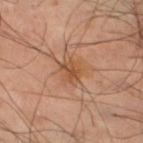<case>
  <biopsy_status>not biopsied; imaged during a skin examination</biopsy_status>
  <lesion_size>
    <long_diameter_mm_approx>3.0</long_diameter_mm_approx>
  </lesion_size>
  <image>
    <source>total-body photography crop</source>
    <field_of_view_mm>15</field_of_view_mm>
  </image>
  <lighting>cross-polarized</lighting>
  <site>left lower leg</site>
  <automated_metrics>
    <area_mm2_approx>5.5</area_mm2_approx>
    <eccentricity>0.45</eccentricity>
    <shape_asymmetry>0.25</shape_asymmetry>
    <border_irregularity_0_10>2.0</border_irregularity_0_10>
    <color_variation_0_10>3.5</color_variation_0_10>
    <peripheral_color_asymmetry>1.0</peripheral_color_asymmetry>
    <lesion_detection_confidence_0_100>100</lesion_detection_confidence_0_100>
  </automated_metrics>
  <patient>
    <sex>male</sex>
    <age_approx>50</age_approx>
  </patient>
</case>